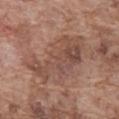Impression:
Part of a total-body skin-imaging series; this lesion was reviewed on a skin check and was not flagged for biopsy.
Clinical summary:
A 15 mm close-up extracted from a 3D total-body photography capture. Captured under white-light illumination. A male subject, aged 73 to 77. Measured at roughly 8 mm in maximum diameter. The total-body-photography lesion software estimated a footprint of about 27 mm², a shape eccentricity near 0.8, and two-axis asymmetry of about 0.35. The lesion is located on the abdomen.